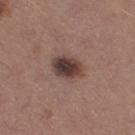workup: imaged on a skin check; not biopsied | patient: female, about 20 years old | tile lighting: white-light illumination | location: the right thigh | size: about 4 mm | automated metrics: a lesion area of about 9 mm², an eccentricity of roughly 0.7, and a symmetry-axis asymmetry near 0.2; a mean CIELAB color near L≈39 a*≈17 b*≈20, a lesion–skin lightness drop of about 14, and a normalized lesion–skin contrast near 11; a border-irregularity index near 2/10, a color-variation rating of about 7/10, and peripheral color asymmetry of about 2; a classifier nevus-likeness of about 80/100 and a lesion-detection confidence of about 100/100 | image source: total-body-photography crop, ~15 mm field of view.